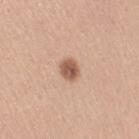anatomic site: the right upper arm | TBP lesion metrics: a lesion area of about 4.5 mm², an outline eccentricity of about 0.65 (0 = round, 1 = elongated), and a symmetry-axis asymmetry near 0.2; a mean CIELAB color near L≈57 a*≈21 b*≈30, a lesion–skin lightness drop of about 14, and a normalized lesion–skin contrast near 9.5; a color-variation rating of about 4/10 and radial color variation of about 1.5; a detector confidence of about 100 out of 100 that the crop contains a lesion | patient: female, aged approximately 30 | acquisition: total-body-photography crop, ~15 mm field of view.